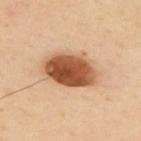<case>
<biopsy_status>not biopsied; imaged during a skin examination</biopsy_status>
<image>
  <source>total-body photography crop</source>
  <field_of_view_mm>15</field_of_view_mm>
</image>
<patient>
  <sex>male</sex>
  <age_approx>65</age_approx>
</patient>
<site>upper back</site>
</case>